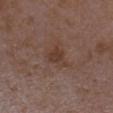| key | value |
|---|---|
| workup | catalogued during a skin exam; not biopsied |
| subject | female, roughly 35 years of age |
| location | the left forearm |
| image source | 15 mm crop, total-body photography |
| image-analysis metrics | a border-irregularity index near 4.5/10, a within-lesion color-variation index near 2/10, and peripheral color asymmetry of about 0.5 |
| diameter | ≈3.5 mm |
| illumination | white-light |